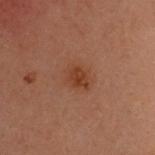The lesion was photographed on a routine skin check and not biopsied; there is no pathology result. Cropped from a total-body skin-imaging series; the visible field is about 15 mm. The subject is a female roughly 40 years of age. The total-body-photography lesion software estimated an area of roughly 7 mm², an outline eccentricity of about 0.55 (0 = round, 1 = elongated), and a symmetry-axis asymmetry near 0.2. It also reported a mean CIELAB color near L≈33 a*≈20 b*≈26, roughly 6 lightness units darker than nearby skin, and a normalized border contrast of about 6.5. It also reported border irregularity of about 2 on a 0–10 scale, internal color variation of about 2.5 on a 0–10 scale, and peripheral color asymmetry of about 1. Located on the head or neck. Approximately 3 mm at its widest. Captured under cross-polarized illumination.